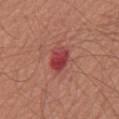Q: Is there a histopathology result?
A: no biopsy performed (imaged during a skin exam)
Q: Illumination type?
A: white-light
Q: What kind of image is this?
A: 15 mm crop, total-body photography
Q: Lesion location?
A: the chest
Q: What are the patient's age and sex?
A: male, in their mid-60s
Q: Automated lesion metrics?
A: an area of roughly 6.5 mm² and an outline eccentricity of about 0.75 (0 = round, 1 = elongated); a lesion color around L≈43 a*≈35 b*≈24 in CIELAB and a normalized lesion–skin contrast near 9; internal color variation of about 5.5 on a 0–10 scale and radial color variation of about 1.5; a nevus-likeness score of about 0/100 and lesion-presence confidence of about 100/100
Q: What is the lesion's diameter?
A: ≈3.5 mm